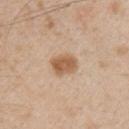Recorded during total-body skin imaging; not selected for excision or biopsy.
The tile uses white-light illumination.
A male patient approximately 50 years of age.
The total-body-photography lesion software estimated an average lesion color of about L≈59 a*≈19 b*≈34 (CIELAB), about 12 CIELAB-L* units darker than the surrounding skin, and a normalized lesion–skin contrast near 8.5. The analysis additionally found a nevus-likeness score of about 95/100.
A lesion tile, about 15 mm wide, cut from a 3D total-body photograph.
The lesion is on the front of the torso.
Measured at roughly 3.5 mm in maximum diameter.